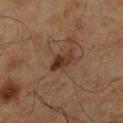Impression:
No biopsy was performed on this lesion — it was imaged during a full skin examination and was not determined to be concerning.
Clinical summary:
A region of skin cropped from a whole-body photographic capture, roughly 15 mm wide. The tile uses cross-polarized illumination. The lesion-visualizer software estimated a lesion color around L≈26 a*≈16 b*≈23 in CIELAB and roughly 8 lightness units darker than nearby skin. The analysis additionally found border irregularity of about 3.5 on a 0–10 scale and a color-variation rating of about 4.5/10. And it measured an automated nevus-likeness rating near 85 out of 100 and lesion-presence confidence of about 100/100. Located on the right lower leg. The lesion's longest dimension is about 2.5 mm. The subject is a male roughly 65 years of age.